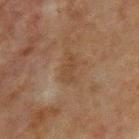Q: Was this lesion biopsied?
A: no biopsy performed (imaged during a skin exam)
Q: Who is the patient?
A: male, aged approximately 65
Q: How was the tile lit?
A: cross-polarized illumination
Q: What did automated image analysis measure?
A: an outline eccentricity of about 0.85 (0 = round, 1 = elongated) and a shape-asymmetry score of about 0.35 (0 = symmetric); a border-irregularity index near 4/10, internal color variation of about 1.5 on a 0–10 scale, and a peripheral color-asymmetry measure near 0.5
Q: How was this image acquired?
A: ~15 mm crop, total-body skin-cancer survey
Q: What is the lesion's diameter?
A: about 3.5 mm
Q: Where on the body is the lesion?
A: the front of the torso